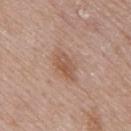No biopsy was performed on this lesion — it was imaged during a full skin examination and was not determined to be concerning.
A lesion tile, about 15 mm wide, cut from a 3D total-body photograph.
A male patient, approximately 75 years of age.
Located on the mid back.
An algorithmic analysis of the crop reported a mean CIELAB color near L≈55 a*≈20 b*≈30, roughly 8 lightness units darker than nearby skin, and a lesion-to-skin contrast of about 6.5 (normalized; higher = more distinct).
Captured under white-light illumination.
The recorded lesion diameter is about 4 mm.